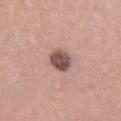workup = total-body-photography surveillance lesion; no biopsy
location = the right thigh
patient = female, in their 40s
acquisition = ~15 mm tile from a whole-body skin photo
tile lighting = white-light
diameter = about 3.5 mm